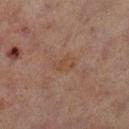The total-body-photography lesion software estimated an average lesion color of about L≈37 a*≈16 b*≈24 (CIELAB) and a lesion-to-skin contrast of about 4.5 (normalized; higher = more distinct).
Located on the leg.
A lesion tile, about 15 mm wide, cut from a 3D total-body photograph.
A male subject in their 70s.
The recorded lesion diameter is about 2.5 mm.
Captured under cross-polarized illumination.